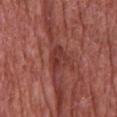{"biopsy_status": "not biopsied; imaged during a skin examination", "site": "chest", "patient": {"sex": "male", "age_approx": 65}, "image": {"source": "total-body photography crop", "field_of_view_mm": 15}}